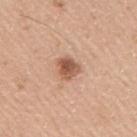Q: Was a biopsy performed?
A: imaged on a skin check; not biopsied
Q: Lesion location?
A: the right upper arm
Q: Who is the patient?
A: male, in their 40s
Q: What lighting was used for the tile?
A: white-light illumination
Q: What kind of image is this?
A: 15 mm crop, total-body photography